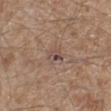Q: Was this lesion biopsied?
A: imaged on a skin check; not biopsied
Q: How was the tile lit?
A: white-light illumination
Q: What is the anatomic site?
A: the left lower leg
Q: What are the patient's age and sex?
A: male, in their mid-60s
Q: What did automated image analysis measure?
A: a lesion area of about 4 mm², an outline eccentricity of about 0.7 (0 = round, 1 = elongated), and a symmetry-axis asymmetry near 0.3; a nevus-likeness score of about 0/100 and a detector confidence of about 90 out of 100 that the crop contains a lesion
Q: What kind of image is this?
A: 15 mm crop, total-body photography